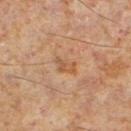biopsy_status: not biopsied; imaged during a skin examination
patient:
  sex: male
  age_approx: 60
image:
  source: total-body photography crop
  field_of_view_mm: 15
site: left lower leg